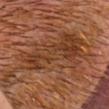Captured during whole-body skin photography for melanoma surveillance; the lesion was not biopsied. Measured at roughly 11 mm in maximum diameter. This image is a 15 mm lesion crop taken from a total-body photograph. Automated tile analysis of the lesion measured a lesion area of about 37 mm² and an eccentricity of roughly 0.85. The analysis additionally found a lesion color around L≈39 a*≈22 b*≈32 in CIELAB and a lesion-to-skin contrast of about 7 (normalized; higher = more distinct). The analysis additionally found a lesion-detection confidence of about 75/100. Located on the head or neck. A male patient, approximately 30 years of age. This is a cross-polarized tile.